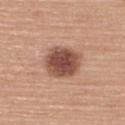  biopsy_status: not biopsied; imaged during a skin examination
  lighting: white-light
  lesion_size:
    long_diameter_mm_approx: 4.5
  site: upper back
  automated_metrics:
    peripheral_color_asymmetry: 1.0
  image:
    source: total-body photography crop
    field_of_view_mm: 15
  patient:
    sex: female
    age_approx: 65